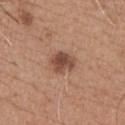Q: Was this lesion biopsied?
A: total-body-photography surveillance lesion; no biopsy
Q: Where on the body is the lesion?
A: the chest
Q: Who is the patient?
A: male, aged approximately 65
Q: What lighting was used for the tile?
A: white-light
Q: What kind of image is this?
A: ~15 mm tile from a whole-body skin photo
Q: Automated lesion metrics?
A: an eccentricity of roughly 0.55; a border-irregularity index near 2.5/10 and peripheral color asymmetry of about 1
Q: How large is the lesion?
A: ~3 mm (longest diameter)